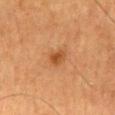The lesion was photographed on a routine skin check and not biopsied; there is no pathology result.
A close-up tile cropped from a whole-body skin photograph, about 15 mm across.
On the back.
A male subject, aged around 60.
Measured at roughly 2.5 mm in maximum diameter.
This is a cross-polarized tile.